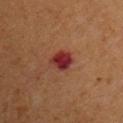Q: Was a biopsy performed?
A: no biopsy performed (imaged during a skin exam)
Q: Where on the body is the lesion?
A: the chest
Q: Automated lesion metrics?
A: a footprint of about 6.5 mm² and two-axis asymmetry of about 0.25; a mean CIELAB color near L≈30 a*≈28 b*≈23 and a lesion–skin lightness drop of about 11; a border-irregularity index near 2.5/10, internal color variation of about 5 on a 0–10 scale, and radial color variation of about 1.5; a classifier nevus-likeness of about 0/100 and a lesion-detection confidence of about 100/100
Q: Illumination type?
A: cross-polarized
Q: What are the patient's age and sex?
A: male, aged 53 to 57
Q: Lesion size?
A: ~3.5 mm (longest diameter)
Q: What kind of image is this?
A: ~15 mm tile from a whole-body skin photo